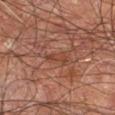workup: no biopsy performed (imaged during a skin exam) | site: the right leg | illumination: cross-polarized illumination | acquisition: ~15 mm tile from a whole-body skin photo | size: about 2.5 mm | automated lesion analysis: a lesion color around L≈41 a*≈23 b*≈29 in CIELAB, about 6 CIELAB-L* units darker than the surrounding skin, and a lesion-to-skin contrast of about 5 (normalized; higher = more distinct) | patient: male, about 60 years old.